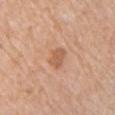image: 15 mm crop, total-body photography | illumination: white-light | TBP lesion metrics: a lesion area of about 4 mm², an outline eccentricity of about 0.7 (0 = round, 1 = elongated), and two-axis asymmetry of about 0.25; internal color variation of about 1 on a 0–10 scale and peripheral color asymmetry of about 0.5 | size: about 2.5 mm | subject: female, in their 70s | site: the chest.